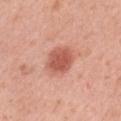Q: Was this lesion biopsied?
A: imaged on a skin check; not biopsied
Q: Patient demographics?
A: female, in their mid-50s
Q: Automated lesion metrics?
A: a lesion color around L≈56 a*≈29 b*≈31 in CIELAB and a lesion-to-skin contrast of about 8 (normalized; higher = more distinct); border irregularity of about 1.5 on a 0–10 scale and radial color variation of about 0.5
Q: Where on the body is the lesion?
A: the left thigh
Q: What kind of image is this?
A: total-body-photography crop, ~15 mm field of view
Q: Illumination type?
A: white-light illumination